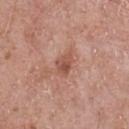Q: Is there a histopathology result?
A: catalogued during a skin exam; not biopsied
Q: How was this image acquired?
A: total-body-photography crop, ~15 mm field of view
Q: What are the patient's age and sex?
A: male, roughly 70 years of age
Q: What is the lesion's diameter?
A: ≈3 mm
Q: Automated lesion metrics?
A: a lesion area of about 5 mm² and a shape eccentricity near 0.8; a mean CIELAB color near L≈53 a*≈24 b*≈29 and roughly 9 lightness units darker than nearby skin; a border-irregularity index near 2.5/10 and radial color variation of about 1.5; a nevus-likeness score of about 0/100 and lesion-presence confidence of about 100/100
Q: Lesion location?
A: the chest
Q: Illumination type?
A: white-light